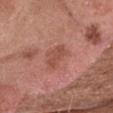Clinical impression:
The lesion was tiled from a total-body skin photograph and was not biopsied.
Acquisition and patient details:
The total-body-photography lesion software estimated a lesion area of about 5 mm² and a shape eccentricity near 0.75. And it measured a lesion color around L≈50 a*≈25 b*≈28 in CIELAB and a normalized lesion–skin contrast near 6. And it measured border irregularity of about 3 on a 0–10 scale, internal color variation of about 1.5 on a 0–10 scale, and peripheral color asymmetry of about 0.5. The patient is a male about 75 years old. Cropped from a total-body skin-imaging series; the visible field is about 15 mm. Located on the head or neck.